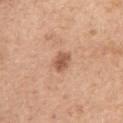Recorded during total-body skin imaging; not selected for excision or biopsy. Imaged with white-light lighting. This image is a 15 mm lesion crop taken from a total-body photograph. The lesion is on the right upper arm. Longest diameter approximately 2.5 mm. A female subject, aged 63–67.